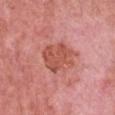Part of a total-body skin-imaging series; this lesion was reviewed on a skin check and was not flagged for biopsy.
The lesion is on the chest.
The subject is a female aged around 40.
A 15 mm close-up extracted from a 3D total-body photography capture.